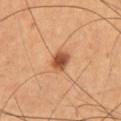Clinical impression: This lesion was catalogued during total-body skin photography and was not selected for biopsy. Image and clinical context: Automated image analysis of the tile measured an area of roughly 4.5 mm², an outline eccentricity of about 0.75 (0 = round, 1 = elongated), and two-axis asymmetry of about 0.15. And it measured a lesion color around L≈46 a*≈23 b*≈33 in CIELAB, about 14 CIELAB-L* units darker than the surrounding skin, and a normalized border contrast of about 10. It also reported a border-irregularity rating of about 1.5/10 and a peripheral color-asymmetry measure near 1. About 3 mm across. The subject is a male aged 58–62. This is a cross-polarized tile. A 15 mm close-up extracted from a 3D total-body photography capture. Located on the right thigh.